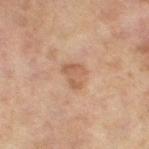Assessment:
Part of a total-body skin-imaging series; this lesion was reviewed on a skin check and was not flagged for biopsy.
Context:
A 15 mm close-up tile from a total-body photography series done for melanoma screening. A female subject aged 58–62. The lesion is on the left thigh.